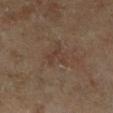No biopsy was performed on this lesion — it was imaged during a full skin examination and was not determined to be concerning.
The subject is a female approximately 65 years of age.
The tile uses cross-polarized illumination.
The lesion is on the leg.
The recorded lesion diameter is about 2.5 mm.
This image is a 15 mm lesion crop taken from a total-body photograph.